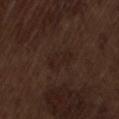{"biopsy_status": "not biopsied; imaged during a skin examination", "site": "lower back", "image": {"source": "total-body photography crop", "field_of_view_mm": 15}, "patient": {"sex": "male", "age_approx": 70}}